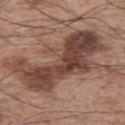A region of skin cropped from a whole-body photographic capture, roughly 15 mm wide.
The lesion's longest dimension is about 13 mm.
A male patient, aged 63–67.
From the arm.
Automated tile analysis of the lesion measured a footprint of about 49 mm², an eccentricity of roughly 0.9, and a symmetry-axis asymmetry near 0.45. It also reported a mean CIELAB color near L≈44 a*≈19 b*≈24, roughly 13 lightness units darker than nearby skin, and a normalized lesion–skin contrast near 9.5. And it measured a border-irregularity index near 8/10.
The tile uses white-light illumination.